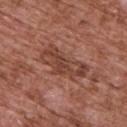follow-up: no biopsy performed (imaged during a skin exam)
automated metrics: an average lesion color of about L≈43 a*≈23 b*≈27 (CIELAB) and roughly 9 lightness units darker than nearby skin; a color-variation rating of about 4.5/10 and radial color variation of about 1.5
lesion diameter: ≈6 mm
image source: total-body-photography crop, ~15 mm field of view
subject: male, in their mid-70s
illumination: white-light illumination
location: the upper back